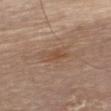Impression: Part of a total-body skin-imaging series; this lesion was reviewed on a skin check and was not flagged for biopsy. Clinical summary: A female subject roughly 65 years of age. The lesion is on the front of the torso. This is a white-light tile. Automated image analysis of the tile measured a lesion area of about 5.5 mm² and a shape-asymmetry score of about 0.25 (0 = symmetric). The software also gave a lesion color around L≈50 a*≈18 b*≈30 in CIELAB, about 7 CIELAB-L* units darker than the surrounding skin, and a normalized lesion–skin contrast near 6. Cropped from a total-body skin-imaging series; the visible field is about 15 mm. The recorded lesion diameter is about 4 mm.